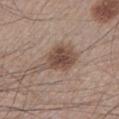| feature | finding |
|---|---|
| workup | catalogued during a skin exam; not biopsied |
| automated metrics | a lesion color around L≈48 a*≈16 b*≈24 in CIELAB and roughly 12 lightness units darker than nearby skin; a within-lesion color-variation index near 4.5/10 and radial color variation of about 1.5; a nevus-likeness score of about 90/100 and a detector confidence of about 100 out of 100 that the crop contains a lesion |
| tile lighting | white-light illumination |
| diameter | ~5.5 mm (longest diameter) |
| body site | the left lower leg |
| acquisition | ~15 mm tile from a whole-body skin photo |
| patient | male, about 45 years old |